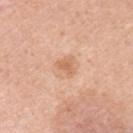Q: What are the patient's age and sex?
A: female, aged 28–32
Q: Lesion location?
A: the left upper arm
Q: What kind of image is this?
A: ~15 mm crop, total-body skin-cancer survey
Q: What did pathology find?
A: an atypical intraepithelial melanocytic proliferation — an indeterminate (borderline) lesion